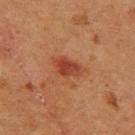- follow-up · imaged on a skin check; not biopsied
- patient · female, aged around 50
- automated lesion analysis · a mean CIELAB color near L≈34 a*≈25 b*≈29 and a normalized lesion–skin contrast near 8; a classifier nevus-likeness of about 100/100
- diameter · ~3.5 mm (longest diameter)
- body site · the left thigh
- illumination · cross-polarized illumination
- imaging modality · ~15 mm tile from a whole-body skin photo